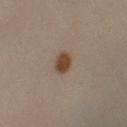  biopsy_status: not biopsied; imaged during a skin examination
  image:
    source: total-body photography crop
    field_of_view_mm: 15
  patient:
    sex: female
    age_approx: 30
  site: left lower leg
  automated_metrics:
    area_mm2_approx: 5.0
    eccentricity: 0.65
    shape_asymmetry: 0.15
    vs_skin_contrast_norm: 10.0
    nevus_likeness_0_100: 100
  lesion_size:
    long_diameter_mm_approx: 3.0